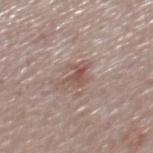Assessment: Imaged during a routine full-body skin examination; the lesion was not biopsied and no histopathology is available. Context: A male subject, aged 73–77. Cropped from a whole-body photographic skin survey; the tile spans about 15 mm. The lesion is on the mid back.